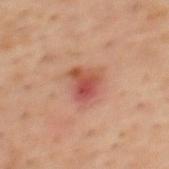follow-up = no biopsy performed (imaged during a skin exam)
illumination = cross-polarized
site = the mid back
patient = male, aged 53–57
image-analysis metrics = a lesion–skin lightness drop of about 11; border irregularity of about 4 on a 0–10 scale; a detector confidence of about 100 out of 100 that the crop contains a lesion
image = ~15 mm crop, total-body skin-cancer survey